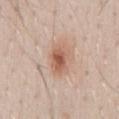Assessment:
Captured during whole-body skin photography for melanoma surveillance; the lesion was not biopsied.
Clinical summary:
The lesion's longest dimension is about 3.5 mm. The lesion is located on the chest. A 15 mm close-up extracted from a 3D total-body photography capture. Automated image analysis of the tile measured border irregularity of about 1.5 on a 0–10 scale, internal color variation of about 4.5 on a 0–10 scale, and peripheral color asymmetry of about 1. This is a white-light tile. A male patient in their 30s.